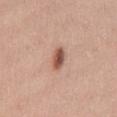Notes:
* workup · no biopsy performed (imaged during a skin exam)
* lighting · white-light
* diameter · about 3 mm
* patient · female, aged 33–37
* anatomic site · the mid back
* acquisition · ~15 mm crop, total-body skin-cancer survey
* automated metrics · a nevus-likeness score of about 95/100 and lesion-presence confidence of about 100/100